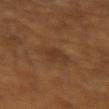Impression: The lesion was tiled from a total-body skin photograph and was not biopsied. Context: A lesion tile, about 15 mm wide, cut from a 3D total-body photograph. A male patient, about 65 years old. The tile uses cross-polarized illumination. The lesion-visualizer software estimated an area of roughly 3 mm², an eccentricity of roughly 0.85, and a symmetry-axis asymmetry near 0.35. About 3 mm across.